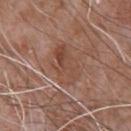Impression:
No biopsy was performed on this lesion — it was imaged during a full skin examination and was not determined to be concerning.
Acquisition and patient details:
Measured at roughly 5 mm in maximum diameter. From the upper back. This is a white-light tile. A 15 mm crop from a total-body photograph taken for skin-cancer surveillance. The patient is a male aged 58 to 62.